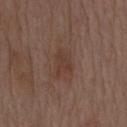<lesion>
  <biopsy_status>not biopsied; imaged during a skin examination</biopsy_status>
  <site>mid back</site>
  <lighting>white-light</lighting>
  <automated_metrics>
    <color_variation_0_10>1.5</color_variation_0_10>
    <peripheral_color_asymmetry>0.5</peripheral_color_asymmetry>
    <nevus_likeness_0_100>25</nevus_likeness_0_100>
    <lesion_detection_confidence_0_100>100</lesion_detection_confidence_0_100>
  </automated_metrics>
  <image>
    <source>total-body photography crop</source>
    <field_of_view_mm>15</field_of_view_mm>
  </image>
  <patient>
    <sex>male</sex>
    <age_approx>70</age_approx>
  </patient>
</lesion>